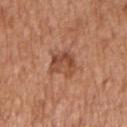The lesion was tiled from a total-body skin photograph and was not biopsied.
A female patient, about 75 years old.
About 3.5 mm across.
This image is a 15 mm lesion crop taken from a total-body photograph.
The lesion is located on the right upper arm.
Imaged with white-light lighting.